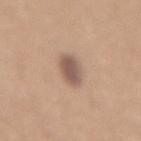notes = catalogued during a skin exam; not biopsied | patient = female, aged 33 to 37 | lesion diameter = ≈3.5 mm | illumination = white-light illumination | image = total-body-photography crop, ~15 mm field of view | site = the lower back | automated metrics = about 13 CIELAB-L* units darker than the surrounding skin; a border-irregularity index near 2/10, a within-lesion color-variation index near 3/10, and radial color variation of about 1; a classifier nevus-likeness of about 85/100.